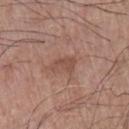Case summary:
- workup · no biopsy performed (imaged during a skin exam)
- image source · 15 mm crop, total-body photography
- illumination · white-light
- patient · male, about 65 years old
- automated metrics · an eccentricity of roughly 0.8; a border-irregularity rating of about 4.5/10, internal color variation of about 0.5 on a 0–10 scale, and peripheral color asymmetry of about 0.5; an automated nevus-likeness rating near 0 out of 100 and lesion-presence confidence of about 100/100
- lesion size · about 3 mm
- site · the left forearm